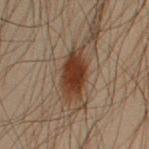anatomic site: the right upper arm
acquisition: total-body-photography crop, ~15 mm field of view
patient: male, aged approximately 50
automated metrics: border irregularity of about 2.5 on a 0–10 scale, a color-variation rating of about 3/10, and a peripheral color-asymmetry measure near 1; lesion-presence confidence of about 100/100
lighting: cross-polarized